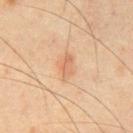Findings:
• notes — no biopsy performed (imaged during a skin exam)
• patient — male, aged 43 to 47
• location — the chest
• diameter — about 3 mm
• tile lighting — cross-polarized
• image — 15 mm crop, total-body photography